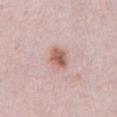The lesion was photographed on a routine skin check and not biopsied; there is no pathology result. This image is a 15 mm lesion crop taken from a total-body photograph. The lesion is located on the chest. The patient is a male aged approximately 25. Longest diameter approximately 3 mm.